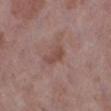The lesion was tiled from a total-body skin photograph and was not biopsied. From the left lower leg. This is a white-light tile. A region of skin cropped from a whole-body photographic capture, roughly 15 mm wide. Automated tile analysis of the lesion measured a shape eccentricity near 0.8. And it measured a mean CIELAB color near L≈47 a*≈20 b*≈23, a lesion–skin lightness drop of about 8, and a lesion-to-skin contrast of about 6 (normalized; higher = more distinct). A female subject, aged around 70.